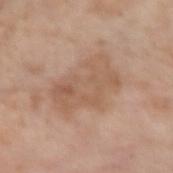The lesion was photographed on a routine skin check and not biopsied; there is no pathology result. The recorded lesion diameter is about 6.5 mm. A region of skin cropped from a whole-body photographic capture, roughly 15 mm wide. Captured under white-light illumination. From the right upper arm. A female patient roughly 85 years of age or older. Automated image analysis of the tile measured an eccentricity of roughly 0.8. The analysis additionally found a border-irregularity index near 4.5/10, a color-variation rating of about 3.5/10, and a peripheral color-asymmetry measure near 1. The software also gave a nevus-likeness score of about 0/100 and lesion-presence confidence of about 100/100.